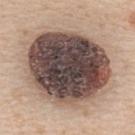Recorded during total-body skin imaging; not selected for excision or biopsy.
On the upper back.
Imaged with white-light lighting.
An algorithmic analysis of the crop reported an average lesion color of about L≈45 a*≈14 b*≈19 (CIELAB). It also reported border irregularity of about 1 on a 0–10 scale, a within-lesion color-variation index near 9/10, and a peripheral color-asymmetry measure near 2.5.
Cropped from a whole-body photographic skin survey; the tile spans about 15 mm.
The subject is a female aged approximately 50.
Longest diameter approximately 10 mm.